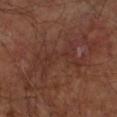biopsy status: imaged on a skin check; not biopsied
anatomic site: the right upper arm
image-analysis metrics: an eccentricity of roughly 0.9; an average lesion color of about L≈30 a*≈20 b*≈23 (CIELAB), a lesion–skin lightness drop of about 4, and a lesion-to-skin contrast of about 5 (normalized; higher = more distinct); border irregularity of about 9 on a 0–10 scale, internal color variation of about 1.5 on a 0–10 scale, and peripheral color asymmetry of about 0.5
lesion size: about 6.5 mm
imaging modality: ~15 mm crop, total-body skin-cancer survey
lighting: cross-polarized
subject: male, in their mid- to late 60s